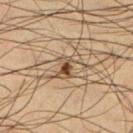No biopsy was performed on this lesion — it was imaged during a full skin examination and was not determined to be concerning. This is a cross-polarized tile. Cropped from a total-body skin-imaging series; the visible field is about 15 mm. Longest diameter approximately 3.5 mm. The subject is a male roughly 35 years of age. On the left lower leg.